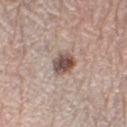Imaged during a routine full-body skin examination; the lesion was not biopsied and no histopathology is available.
A 15 mm crop from a total-body photograph taken for skin-cancer surveillance.
The lesion is located on the right lower leg.
Longest diameter approximately 3 mm.
The total-body-photography lesion software estimated a lesion color around L≈50 a*≈16 b*≈22 in CIELAB, a lesion–skin lightness drop of about 16, and a lesion-to-skin contrast of about 10.5 (normalized; higher = more distinct). The analysis additionally found a border-irregularity rating of about 2/10, a within-lesion color-variation index near 4.5/10, and radial color variation of about 1.5.
A female subject, aged around 65.
The tile uses white-light illumination.